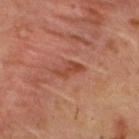Impression:
Recorded during total-body skin imaging; not selected for excision or biopsy.
Clinical summary:
The lesion's longest dimension is about 3.5 mm. Captured under cross-polarized illumination. The subject is a male in their mid- to late 50s. This image is a 15 mm lesion crop taken from a total-body photograph. On the upper back.